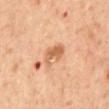Part of a total-body skin-imaging series; this lesion was reviewed on a skin check and was not flagged for biopsy. A 15 mm crop from a total-body photograph taken for skin-cancer surveillance. On the back. Measured at roughly 3.5 mm in maximum diameter. Automated tile analysis of the lesion measured a mean CIELAB color near L≈61 a*≈23 b*≈37 and a normalized lesion–skin contrast near 7. It also reported an automated nevus-likeness rating near 0 out of 100 and a detector confidence of about 100 out of 100 that the crop contains a lesion. A female patient, roughly 45 years of age.